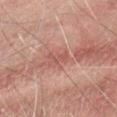Clinical impression:
The lesion was photographed on a routine skin check and not biopsied; there is no pathology result.
Image and clinical context:
Located on the abdomen. The lesion's longest dimension is about 2.5 mm. A 15 mm crop from a total-body photograph taken for skin-cancer surveillance. The total-body-photography lesion software estimated roughly 6 lightness units darker than nearby skin and a normalized border contrast of about 5. The analysis additionally found a border-irregularity rating of about 3.5/10, a within-lesion color-variation index near 0/10, and a peripheral color-asymmetry measure near 0. It also reported a classifier nevus-likeness of about 0/100. A male subject roughly 60 years of age. Captured under cross-polarized illumination.